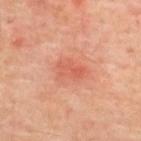Clinical impression:
Part of a total-body skin-imaging series; this lesion was reviewed on a skin check and was not flagged for biopsy.
Background:
An algorithmic analysis of the crop reported a lesion area of about 8 mm², an outline eccentricity of about 0.75 (0 = round, 1 = elongated), and two-axis asymmetry of about 0.25. The analysis additionally found about 7 CIELAB-L* units darker than the surrounding skin. And it measured border irregularity of about 2.5 on a 0–10 scale, internal color variation of about 3.5 on a 0–10 scale, and a peripheral color-asymmetry measure near 1.5. Imaged with cross-polarized lighting. Located on the upper back. This image is a 15 mm lesion crop taken from a total-body photograph. A male patient, in their mid-60s.